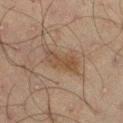workup: no biopsy performed (imaged during a skin exam)
subject: male, approximately 50 years of age
image source: total-body-photography crop, ~15 mm field of view
anatomic site: the right thigh